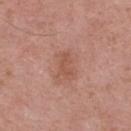workup: catalogued during a skin exam; not biopsied
imaging modality: ~15 mm crop, total-body skin-cancer survey
subject: male, in their mid-60s
diameter: ~2.5 mm (longest diameter)
location: the upper back